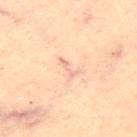Assessment: The lesion was tiled from a total-body skin photograph and was not biopsied. Image and clinical context: The lesion is located on the upper back. A region of skin cropped from a whole-body photographic capture, roughly 15 mm wide. A female subject, about 45 years old.